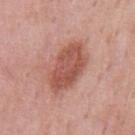Notes:
• image source — ~15 mm tile from a whole-body skin photo
• TBP lesion metrics — a border-irregularity rating of about 2.5/10 and a color-variation rating of about 3.5/10; a nevus-likeness score of about 85/100 and a detector confidence of about 100 out of 100 that the crop contains a lesion
• subject — male, roughly 55 years of age
• illumination — white-light illumination
• site — the chest